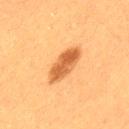follow-up: no biopsy performed (imaged during a skin exam)
lighting: cross-polarized
size: ~5 mm (longest diameter)
automated metrics: a border-irregularity index near 2.5/10; lesion-presence confidence of about 100/100
site: the left thigh
patient: female, aged 38 to 42
image source: ~15 mm tile from a whole-body skin photo